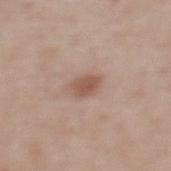Assessment:
The lesion was tiled from a total-body skin photograph and was not biopsied.